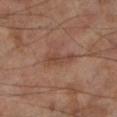No biopsy was performed on this lesion — it was imaged during a full skin examination and was not determined to be concerning. From the left lower leg. Imaged with cross-polarized lighting. The total-body-photography lesion software estimated an area of roughly 4.5 mm², a shape eccentricity near 0.9, and two-axis asymmetry of about 0.35. The software also gave a lesion–skin lightness drop of about 7 and a normalized border contrast of about 6. And it measured a border-irregularity index near 4.5/10 and a peripheral color-asymmetry measure near 0.5. It also reported a nevus-likeness score of about 0/100 and a lesion-detection confidence of about 100/100. A roughly 15 mm field-of-view crop from a total-body skin photograph. About 3.5 mm across. A male patient roughly 70 years of age.